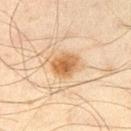This lesion was catalogued during total-body skin photography and was not selected for biopsy. This is a cross-polarized tile. The subject is a male aged 43 to 47. This image is a 15 mm lesion crop taken from a total-body photograph. The lesion's longest dimension is about 3.5 mm. Automated image analysis of the tile measured a border-irregularity index near 1.5/10, internal color variation of about 4.5 on a 0–10 scale, and radial color variation of about 1.5. On the right thigh.